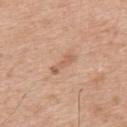This lesion was catalogued during total-body skin photography and was not selected for biopsy. A 15 mm close-up extracted from a 3D total-body photography capture. The lesion is located on the upper back. Imaged with white-light lighting. The recorded lesion diameter is about 3.5 mm. A male subject about 50 years old. An algorithmic analysis of the crop reported a mean CIELAB color near L≈60 a*≈21 b*≈32, roughly 8 lightness units darker than nearby skin, and a normalized border contrast of about 5.5. And it measured a border-irregularity rating of about 4/10 and radial color variation of about 0.